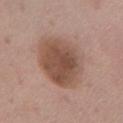Impression:
Imaged during a routine full-body skin examination; the lesion was not biopsied and no histopathology is available.
Context:
Cropped from a whole-body photographic skin survey; the tile spans about 15 mm. This is a white-light tile. Located on the chest. About 6.5 mm across. Automated image analysis of the tile measured a shape-asymmetry score of about 0.1 (0 = symmetric). And it measured a mean CIELAB color near L≈51 a*≈19 b*≈27 and about 12 CIELAB-L* units darker than the surrounding skin. And it measured an automated nevus-likeness rating near 30 out of 100 and lesion-presence confidence of about 100/100. The patient is a female aged 48–52.